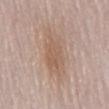Clinical impression: No biopsy was performed on this lesion — it was imaged during a full skin examination and was not determined to be concerning. Image and clinical context: The tile uses white-light illumination. A male subject aged 63–67. The lesion-visualizer software estimated a lesion area of about 16 mm², an eccentricity of roughly 0.9, and two-axis asymmetry of about 0.3. It also reported a mean CIELAB color near L≈58 a*≈16 b*≈28 and roughly 8 lightness units darker than nearby skin. And it measured a border-irregularity index near 4/10 and internal color variation of about 2.5 on a 0–10 scale. The analysis additionally found a classifier nevus-likeness of about 20/100 and a detector confidence of about 100 out of 100 that the crop contains a lesion. About 7.5 mm across. A roughly 15 mm field-of-view crop from a total-body skin photograph. On the mid back.